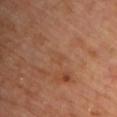Impression: No biopsy was performed on this lesion — it was imaged during a full skin examination and was not determined to be concerning. Background: The total-body-photography lesion software estimated a border-irregularity index near 2/10, a color-variation rating of about 0/10, and peripheral color asymmetry of about 0. It also reported an automated nevus-likeness rating near 0 out of 100 and lesion-presence confidence of about 100/100. Cropped from a whole-body photographic skin survey; the tile spans about 15 mm. This is a cross-polarized tile. Located on the chest. A male patient aged 58 to 62.